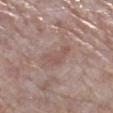workup: imaged on a skin check; not biopsied | acquisition: ~15 mm tile from a whole-body skin photo | location: the right lower leg | patient: female, aged around 70 | automated lesion analysis: two-axis asymmetry of about 0.55; a mean CIELAB color near L≈53 a*≈18 b*≈23, a lesion–skin lightness drop of about 7, and a normalized border contrast of about 5; a nevus-likeness score of about 0/100 and lesion-presence confidence of about 100/100 | lighting: white-light illumination | diameter: ≈4 mm.